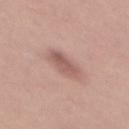{"biopsy_status": "not biopsied; imaged during a skin examination", "patient": {"sex": "female", "age_approx": 25}, "site": "mid back", "image": {"source": "total-body photography crop", "field_of_view_mm": 15}, "lighting": "white-light", "lesion_size": {"long_diameter_mm_approx": 4.0}}